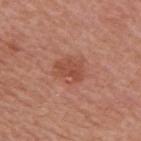Q: Was a biopsy performed?
A: no biopsy performed (imaged during a skin exam)